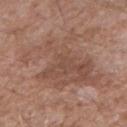workup: no biopsy performed (imaged during a skin exam) | patient: male, in their 50s | tile lighting: white-light | site: the arm | acquisition: 15 mm crop, total-body photography | diameter: ≈9 mm.